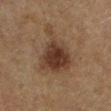Notes:
* workup: catalogued during a skin exam; not biopsied
* imaging modality: 15 mm crop, total-body photography
* lighting: cross-polarized illumination
* patient: male, aged 83 or older
* location: the left lower leg